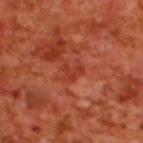workup = no biopsy performed (imaged during a skin exam); lighting = cross-polarized; lesion size = about 2.5 mm; subject = male, aged 68 to 72; image = 15 mm crop, total-body photography; site = the upper back.